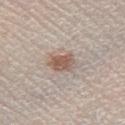Clinical impression: The lesion was photographed on a routine skin check and not biopsied; there is no pathology result. Clinical summary: Approximately 3 mm at its widest. The lesion is located on the right lower leg. A region of skin cropped from a whole-body photographic capture, roughly 15 mm wide. A male patient, aged approximately 35. Captured under white-light illumination.